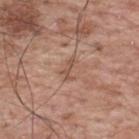Clinical impression:
Imaged during a routine full-body skin examination; the lesion was not biopsied and no histopathology is available.
Background:
Measured at roughly 2.5 mm in maximum diameter. The subject is a male aged approximately 70. Located on the upper back. A lesion tile, about 15 mm wide, cut from a 3D total-body photograph.